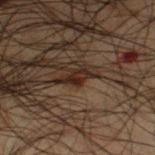notes — total-body-photography surveillance lesion; no biopsy
lesion diameter — about 2.5 mm
image source — ~15 mm tile from a whole-body skin photo
patient — male, aged around 50
location — the left thigh